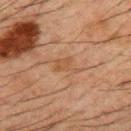<tbp_lesion>
  <biopsy_status>not biopsied; imaged during a skin examination</biopsy_status>
  <patient>
    <sex>male</sex>
    <age_approx>50</age_approx>
  </patient>
  <lesion_size>
    <long_diameter_mm_approx>3.0</long_diameter_mm_approx>
  </lesion_size>
  <site>mid back</site>
  <image>
    <source>total-body photography crop</source>
    <field_of_view_mm>15</field_of_view_mm>
  </image>
</tbp_lesion>